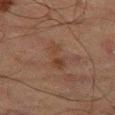Clinical impression: This lesion was catalogued during total-body skin photography and was not selected for biopsy. Background: On the right thigh. The patient is a male about 65 years old. A region of skin cropped from a whole-body photographic capture, roughly 15 mm wide.